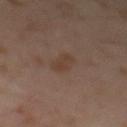Notes:
– biopsy status: imaged on a skin check; not biopsied
– TBP lesion metrics: lesion-presence confidence of about 100/100
– image source: ~15 mm tile from a whole-body skin photo
– lesion diameter: about 3.5 mm
– tile lighting: cross-polarized illumination
– subject: male, aged approximately 65